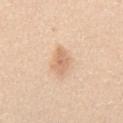<case>
  <biopsy_status>not biopsied; imaged during a skin examination</biopsy_status>
  <image>
    <source>total-body photography crop</source>
    <field_of_view_mm>15</field_of_view_mm>
  </image>
  <lighting>white-light</lighting>
  <patient>
    <sex>male</sex>
    <age_approx>30</age_approx>
  </patient>
  <automated_metrics>
    <area_mm2_approx>5.5</area_mm2_approx>
    <eccentricity>0.85</eccentricity>
    <shape_asymmetry>0.25</shape_asymmetry>
    <cielab_L>69</cielab_L>
    <cielab_a>20</cielab_a>
    <cielab_b>34</cielab_b>
    <vs_skin_darker_L>10.0</vs_skin_darker_L>
    <vs_skin_contrast_norm>6.5</vs_skin_contrast_norm>
    <border_irregularity_0_10>3.0</border_irregularity_0_10>
    <peripheral_color_asymmetry>0.5</peripheral_color_asymmetry>
    <lesion_detection_confidence_0_100>100</lesion_detection_confidence_0_100>
  </automated_metrics>
  <site>abdomen</site>
  <lesion_size>
    <long_diameter_mm_approx>3.5</long_diameter_mm_approx>
  </lesion_size>
</case>